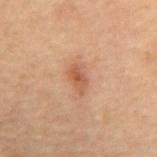biopsy_status: not biopsied; imaged during a skin examination
lesion_size:
  long_diameter_mm_approx: 3.0
patient:
  sex: male
  age_approx: 70
site: mid back
lighting: cross-polarized
image:
  source: total-body photography crop
  field_of_view_mm: 15